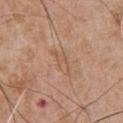The lesion was photographed on a routine skin check and not biopsied; there is no pathology result.
Automated tile analysis of the lesion measured a footprint of about 4.5 mm² and a shape eccentricity near 0.9. The software also gave a within-lesion color-variation index near 2/10.
A male patient, aged around 65.
A 15 mm close-up extracted from a 3D total-body photography capture.
This is a white-light tile.
The lesion is on the chest.